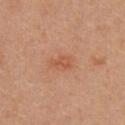follow-up: no biopsy performed (imaged during a skin exam)
site: the chest
TBP lesion metrics: an outline eccentricity of about 0.8 (0 = round, 1 = elongated) and two-axis asymmetry of about 0.5; a lesion color around L≈55 a*≈27 b*≈34 in CIELAB, a lesion–skin lightness drop of about 7, and a normalized lesion–skin contrast near 5.5; a border-irregularity rating of about 4.5/10, a color-variation rating of about 1.5/10, and peripheral color asymmetry of about 0.5; a classifier nevus-likeness of about 0/100
lesion diameter: about 2.5 mm
patient: female, in their mid- to late 20s
image source: ~15 mm crop, total-body skin-cancer survey
lighting: white-light illumination